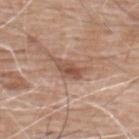{"biopsy_status": "not biopsied; imaged during a skin examination", "site": "back", "image": {"source": "total-body photography crop", "field_of_view_mm": 15}, "lesion_size": {"long_diameter_mm_approx": 3.0}, "automated_metrics": {"area_mm2_approx": 4.5, "shape_asymmetry": 0.25, "border_irregularity_0_10": 3.0, "color_variation_0_10": 3.5, "peripheral_color_asymmetry": 1.0}, "lighting": "white-light", "patient": {"sex": "male", "age_approx": 60}}